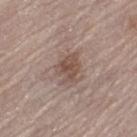workup — total-body-photography surveillance lesion; no biopsy | patient — female, about 65 years old | TBP lesion metrics — an area of roughly 7 mm², an outline eccentricity of about 0.65 (0 = round, 1 = elongated), and two-axis asymmetry of about 0.3; a border-irregularity index near 3.5/10 and a peripheral color-asymmetry measure near 1; lesion-presence confidence of about 100/100 | location — the left thigh | lighting — white-light illumination | diameter — ≈4 mm | imaging modality — ~15 mm tile from a whole-body skin photo.